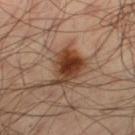Assessment: No biopsy was performed on this lesion — it was imaged during a full skin examination and was not determined to be concerning.